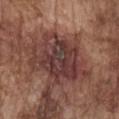| feature | finding |
|---|---|
| workup | total-body-photography surveillance lesion; no biopsy |
| subject | male, in their mid-70s |
| anatomic site | the chest |
| lighting | white-light |
| imaging modality | 15 mm crop, total-body photography |
| automated metrics | an eccentricity of roughly 0.65; an average lesion color of about L≈37 a*≈21 b*≈21 (CIELAB), roughly 11 lightness units darker than nearby skin, and a normalized border contrast of about 10 |